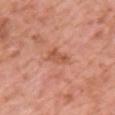Acquisition and patient details:
On the chest. A female subject in their 40s. A close-up tile cropped from a whole-body skin photograph, about 15 mm across.